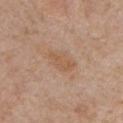{
  "biopsy_status": "not biopsied; imaged during a skin examination",
  "lesion_size": {
    "long_diameter_mm_approx": 4.0
  },
  "site": "chest",
  "automated_metrics": {
    "area_mm2_approx": 7.5,
    "eccentricity": 0.8,
    "shape_asymmetry": 0.15,
    "cielab_L": 56,
    "cielab_a": 18,
    "cielab_b": 32,
    "vs_skin_darker_L": 6.0,
    "vs_skin_contrast_norm": 5.5,
    "border_irregularity_0_10": 2.0,
    "color_variation_0_10": 2.0,
    "peripheral_color_asymmetry": 0.5
  },
  "lighting": "white-light",
  "patient": {
    "sex": "male",
    "age_approx": 60
  },
  "image": {
    "source": "total-body photography crop",
    "field_of_view_mm": 15
  }
}